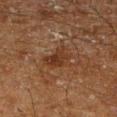This lesion was catalogued during total-body skin photography and was not selected for biopsy.
The subject is a male aged around 60.
Automated image analysis of the tile measured an automated nevus-likeness rating near 5 out of 100 and a detector confidence of about 100 out of 100 that the crop contains a lesion.
This image is a 15 mm lesion crop taken from a total-body photograph.
The lesion is on the right lower leg.
The tile uses cross-polarized illumination.
About 4 mm across.